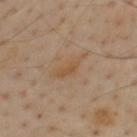Q: Was this lesion biopsied?
A: catalogued during a skin exam; not biopsied
Q: What are the patient's age and sex?
A: male, in their mid- to late 50s
Q: What is the lesion's diameter?
A: ≈4.5 mm
Q: What kind of image is this?
A: ~15 mm crop, total-body skin-cancer survey
Q: What is the anatomic site?
A: the mid back
Q: What lighting was used for the tile?
A: cross-polarized illumination
Q: Automated lesion metrics?
A: a shape eccentricity near 0.9 and a symmetry-axis asymmetry near 0.4; border irregularity of about 4.5 on a 0–10 scale, a color-variation rating of about 1.5/10, and peripheral color asymmetry of about 0.5; a classifier nevus-likeness of about 0/100 and a detector confidence of about 100 out of 100 that the crop contains a lesion